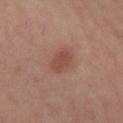Imaged during a routine full-body skin examination; the lesion was not biopsied and no histopathology is available.
A region of skin cropped from a whole-body photographic capture, roughly 15 mm wide.
A female subject, in their mid-50s.
About 3 mm across.
Located on the leg.